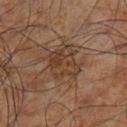Q: Was a biopsy performed?
A: total-body-photography surveillance lesion; no biopsy
Q: Patient demographics?
A: male, approximately 60 years of age
Q: How large is the lesion?
A: ≈4 mm
Q: Automated lesion metrics?
A: an area of roughly 12 mm², an outline eccentricity of about 0.5 (0 = round, 1 = elongated), and two-axis asymmetry of about 0.2; a lesion–skin lightness drop of about 7; a border-irregularity index near 2.5/10, internal color variation of about 5 on a 0–10 scale, and peripheral color asymmetry of about 1.5
Q: What lighting was used for the tile?
A: cross-polarized illumination
Q: What is the imaging modality?
A: ~15 mm crop, total-body skin-cancer survey
Q: What is the anatomic site?
A: the left lower leg